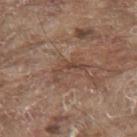The tile uses white-light illumination.
A male subject, roughly 80 years of age.
Longest diameter approximately 4.5 mm.
A 15 mm close-up tile from a total-body photography series done for melanoma screening.
On the back.